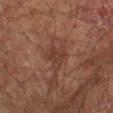follow-up: catalogued during a skin exam; not biopsied | acquisition: total-body-photography crop, ~15 mm field of view | anatomic site: the right upper arm | lighting: cross-polarized illumination | subject: male, about 65 years old | lesion size: ≈3.5 mm.